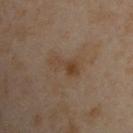Case summary:
• notes · no biopsy performed (imaged during a skin exam)
• tile lighting · cross-polarized
• patient · male, aged approximately 50
• lesion size · ~4 mm (longest diameter)
• site · the left arm
• imaging modality · total-body-photography crop, ~15 mm field of view
• automated lesion analysis · an area of roughly 6 mm², an outline eccentricity of about 0.9 (0 = round, 1 = elongated), and two-axis asymmetry of about 0.3; a mean CIELAB color near L≈32 a*≈12 b*≈24 and a normalized border contrast of about 6.5; a within-lesion color-variation index near 3/10 and a peripheral color-asymmetry measure near 0.5